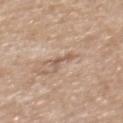Q: Is there a histopathology result?
A: no biopsy performed (imaged during a skin exam)
Q: How was the tile lit?
A: white-light
Q: What are the patient's age and sex?
A: male, aged around 70
Q: What is the imaging modality?
A: ~15 mm crop, total-body skin-cancer survey
Q: Lesion location?
A: the mid back
Q: Automated lesion metrics?
A: a mean CIELAB color near L≈58 a*≈18 b*≈27 and a normalized lesion–skin contrast near 5.5
Q: How large is the lesion?
A: ~2.5 mm (longest diameter)